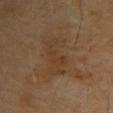Assessment:
Imaged during a routine full-body skin examination; the lesion was not biopsied and no histopathology is available.
Context:
Located on the front of the torso. Cropped from a whole-body photographic skin survey; the tile spans about 15 mm. This is a cross-polarized tile. Automated tile analysis of the lesion measured a mean CIELAB color near L≈35 a*≈15 b*≈29, roughly 5 lightness units darker than nearby skin, and a lesion-to-skin contrast of about 5 (normalized; higher = more distinct). It also reported a border-irregularity rating of about 5.5/10 and a color-variation rating of about 3/10. A male subject aged approximately 70. Approximately 5.5 mm at its widest.